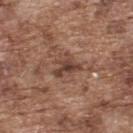Assessment:
Captured during whole-body skin photography for melanoma surveillance; the lesion was not biopsied.
Context:
Automated image analysis of the tile measured a footprint of about 5.5 mm² and an outline eccentricity of about 0.6 (0 = round, 1 = elongated). It also reported a mean CIELAB color near L≈42 a*≈20 b*≈25 and roughly 10 lightness units darker than nearby skin. And it measured a nevus-likeness score of about 10/100 and lesion-presence confidence of about 85/100. Cropped from a whole-body photographic skin survey; the tile spans about 15 mm. A male patient, aged around 75. The lesion is located on the upper back. Imaged with white-light lighting.